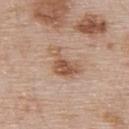– notes — no biopsy performed (imaged during a skin exam)
– subject — male, in their mid- to late 50s
– imaging modality — ~15 mm crop, total-body skin-cancer survey
– anatomic site — the upper back